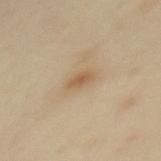Assessment:
The lesion was tiled from a total-body skin photograph and was not biopsied.
Image and clinical context:
Measured at roughly 2.5 mm in maximum diameter. A 15 mm close-up tile from a total-body photography series done for melanoma screening. Captured under cross-polarized illumination. The total-body-photography lesion software estimated a lesion area of about 3 mm², an outline eccentricity of about 0.85 (0 = round, 1 = elongated), and a symmetry-axis asymmetry near 0.3. The software also gave about 9 CIELAB-L* units darker than the surrounding skin and a normalized border contrast of about 6.5. It also reported a border-irregularity index near 3/10, a color-variation rating of about 1/10, and radial color variation of about 0.5. The software also gave a nevus-likeness score of about 25/100. A female patient, aged approximately 40. From the upper back.